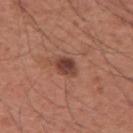Part of a total-body skin-imaging series; this lesion was reviewed on a skin check and was not flagged for biopsy. Longest diameter approximately 3 mm. An algorithmic analysis of the crop reported a border-irregularity index near 1.5/10, a within-lesion color-variation index near 5/10, and peripheral color asymmetry of about 1.5. And it measured a classifier nevus-likeness of about 85/100 and a lesion-detection confidence of about 100/100. Imaged with white-light lighting. A male subject aged 58–62. Cropped from a total-body skin-imaging series; the visible field is about 15 mm. From the right upper arm.